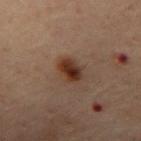The lesion was tiled from a total-body skin photograph and was not biopsied.
A lesion tile, about 15 mm wide, cut from a 3D total-body photograph.
The recorded lesion diameter is about 3 mm.
The subject is a male approximately 60 years of age.
The tile uses cross-polarized illumination.
From the mid back.